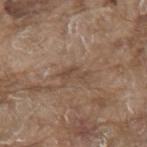Q: Patient demographics?
A: male, roughly 80 years of age
Q: What is the anatomic site?
A: the back
Q: What is the imaging modality?
A: 15 mm crop, total-body photography